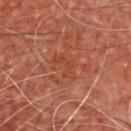The lesion was tiled from a total-body skin photograph and was not biopsied. This image is a 15 mm lesion crop taken from a total-body photograph. This is a cross-polarized tile. A male patient, about 65 years old. The lesion is located on the chest. About 3.5 mm across.